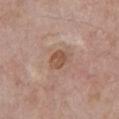| field | value |
|---|---|
| anatomic site | the chest |
| acquisition | ~15 mm tile from a whole-body skin photo |
| size | ~3 mm (longest diameter) |
| illumination | white-light illumination |
| subject | male, aged approximately 60 |
| automated lesion analysis | a mean CIELAB color near L≈52 a*≈20 b*≈29, about 9 CIELAB-L* units darker than the surrounding skin, and a normalized border contrast of about 7.5; a color-variation rating of about 3.5/10 and peripheral color asymmetry of about 1 |